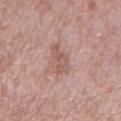The lesion was tiled from a total-body skin photograph and was not biopsied. The lesion is located on the left lower leg. An algorithmic analysis of the crop reported a footprint of about 6 mm² and two-axis asymmetry of about 0.35. The analysis additionally found roughly 8 lightness units darker than nearby skin and a normalized border contrast of about 6. It also reported a within-lesion color-variation index near 2.5/10 and a peripheral color-asymmetry measure near 1. A 15 mm close-up tile from a total-body photography series done for melanoma screening. A male subject roughly 55 years of age.